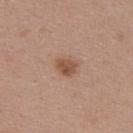{"biopsy_status": "not biopsied; imaged during a skin examination", "site": "upper back", "patient": {"sex": "female", "age_approx": 45}, "image": {"source": "total-body photography crop", "field_of_view_mm": 15}, "lighting": "white-light", "automated_metrics": {"area_mm2_approx": 4.5, "eccentricity": 0.6, "cielab_L": 51, "cielab_a": 20, "cielab_b": 30, "vs_skin_contrast_norm": 7.5, "border_irregularity_0_10": 1.5, "color_variation_0_10": 2.5, "peripheral_color_asymmetry": 1.0, "nevus_likeness_0_100": 90, "lesion_detection_confidence_0_100": 100}, "lesion_size": {"long_diameter_mm_approx": 2.5}}